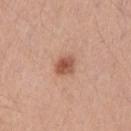A male patient aged 38 to 42. An algorithmic analysis of the crop reported an area of roughly 5 mm². The software also gave a mean CIELAB color near L≈54 a*≈24 b*≈30 and roughly 13 lightness units darker than nearby skin. The analysis additionally found a border-irregularity index near 1.5/10, a within-lesion color-variation index near 4.5/10, and a peripheral color-asymmetry measure near 1.5. The lesion is located on the left upper arm. The lesion's longest dimension is about 2.5 mm. The tile uses white-light illumination. A roughly 15 mm field-of-view crop from a total-body skin photograph.